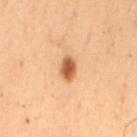Imaged during a routine full-body skin examination; the lesion was not biopsied and no histopathology is available. From the mid back. The subject is a female aged 48 to 52. Automated image analysis of the tile measured a normalized lesion–skin contrast near 10. Cropped from a total-body skin-imaging series; the visible field is about 15 mm. Captured under cross-polarized illumination. Longest diameter approximately 3 mm.